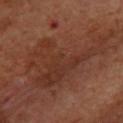biopsy status = catalogued during a skin exam; not biopsied | acquisition = ~15 mm tile from a whole-body skin photo | subject = male, aged 68 to 72 | body site = the upper back.